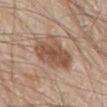Context:
The lesion is located on the abdomen. Approximately 5 mm at its widest. A 15 mm close-up extracted from a 3D total-body photography capture. Captured under white-light illumination. A male subject aged approximately 80. Automated tile analysis of the lesion measured a lesion area of about 18 mm² and a symmetry-axis asymmetry near 0.25.